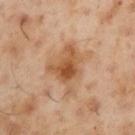follow-up: imaged on a skin check; not biopsied
image source: total-body-photography crop, ~15 mm field of view
subject: male, aged approximately 55
tile lighting: cross-polarized illumination
body site: the left upper arm
size: ≈5.5 mm